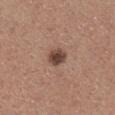Q: Is there a histopathology result?
A: imaged on a skin check; not biopsied
Q: Lesion location?
A: the right lower leg
Q: What kind of image is this?
A: 15 mm crop, total-body photography
Q: What is the lesion's diameter?
A: about 2.5 mm
Q: Patient demographics?
A: male, roughly 60 years of age